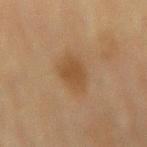Imaged during a routine full-body skin examination; the lesion was not biopsied and no histopathology is available. Cropped from a total-body skin-imaging series; the visible field is about 15 mm. Captured under cross-polarized illumination. An algorithmic analysis of the crop reported a shape eccentricity near 0.85 and a symmetry-axis asymmetry near 0.3. It also reported an average lesion color of about L≈42 a*≈16 b*≈31 (CIELAB) and a lesion-to-skin contrast of about 7 (normalized; higher = more distinct). It also reported a color-variation rating of about 1.5/10 and radial color variation of about 0.5. Located on the mid back. A female patient, aged 78 to 82.